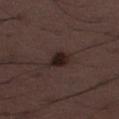No biopsy was performed on this lesion — it was imaged during a full skin examination and was not determined to be concerning. Located on the left thigh. A 15 mm close-up tile from a total-body photography series done for melanoma screening. A male patient, aged around 30. The lesion's longest dimension is about 2.5 mm. The tile uses white-light illumination.